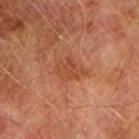follow-up: total-body-photography surveillance lesion; no biopsy